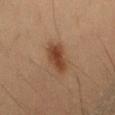The lesion was tiled from a total-body skin photograph and was not biopsied.
The total-body-photography lesion software estimated a lesion color around L≈38 a*≈19 b*≈29 in CIELAB and a normalized lesion–skin contrast near 9. It also reported a border-irregularity index near 2/10, a color-variation rating of about 3/10, and radial color variation of about 1. The analysis additionally found an automated nevus-likeness rating near 100 out of 100 and lesion-presence confidence of about 100/100.
From the back.
A female subject in their 30s.
About 4 mm across.
Imaged with cross-polarized lighting.
A region of skin cropped from a whole-body photographic capture, roughly 15 mm wide.